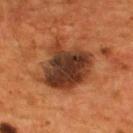| field | value |
|---|---|
| biopsy status | catalogued during a skin exam; not biopsied |
| lighting | cross-polarized illumination |
| imaging modality | ~15 mm crop, total-body skin-cancer survey |
| lesion diameter | ~6 mm (longest diameter) |
| subject | male, in their mid-50s |
| location | the back |